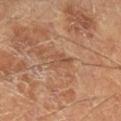follow-up = catalogued during a skin exam; not biopsied
patient = male, aged 58–62
lesion size = about 3 mm
illumination = cross-polarized
imaging modality = 15 mm crop, total-body photography
anatomic site = the right lower leg